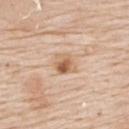biopsy status = catalogued during a skin exam; not biopsied.